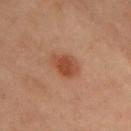No biopsy was performed on this lesion — it was imaged during a full skin examination and was not determined to be concerning.
A female subject aged approximately 55.
A 15 mm crop from a total-body photograph taken for skin-cancer surveillance.
From the chest.